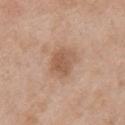About 3.5 mm across. This image is a 15 mm lesion crop taken from a total-body photograph. Captured under white-light illumination. The patient is a male about 50 years old. The lesion is on the chest.